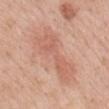Assessment: Imaged during a routine full-body skin examination; the lesion was not biopsied and no histopathology is available. Image and clinical context: Longest diameter approximately 8.5 mm. A 15 mm close-up extracted from a 3D total-body photography capture. On the mid back. The patient is a female aged around 55.